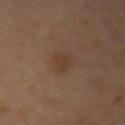This is a cross-polarized tile.
The lesion is on the mid back.
A female patient aged 48–52.
A 15 mm crop from a total-body photograph taken for skin-cancer surveillance.